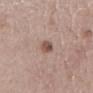Image and clinical context: A male subject, approximately 70 years of age. Located on the mid back. Captured under white-light illumination. Longest diameter approximately 3 mm. Automated image analysis of the tile measured a color-variation rating of about 3/10 and radial color variation of about 1. It also reported an automated nevus-likeness rating near 95 out of 100 and a lesion-detection confidence of about 100/100. A 15 mm close-up extracted from a 3D total-body photography capture.